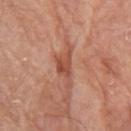Q: Was this lesion biopsied?
A: total-body-photography surveillance lesion; no biopsy
Q: What did automated image analysis measure?
A: border irregularity of about 7.5 on a 0–10 scale, a color-variation rating of about 4/10, and peripheral color asymmetry of about 1.5
Q: Who is the patient?
A: male, aged 78–82
Q: How was the tile lit?
A: white-light
Q: Lesion size?
A: ≈5.5 mm
Q: How was this image acquired?
A: ~15 mm tile from a whole-body skin photo
Q: What is the anatomic site?
A: the arm